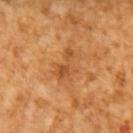Assessment:
The lesion was photographed on a routine skin check and not biopsied; there is no pathology result.
Acquisition and patient details:
This image is a 15 mm lesion crop taken from a total-body photograph. The lesion's longest dimension is about 4 mm. A male patient about 65 years old.